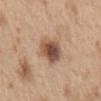workup: imaged on a skin check; not biopsied
image: 15 mm crop, total-body photography
illumination: white-light illumination
anatomic site: the back
image-analysis metrics: roughly 15 lightness units darker than nearby skin; a border-irregularity index near 2/10, internal color variation of about 6 on a 0–10 scale, and a peripheral color-asymmetry measure near 1.5
diameter: ≈4 mm
subject: male, approximately 70 years of age